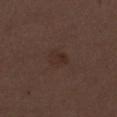The lesion was photographed on a routine skin check and not biopsied; there is no pathology result. Automated tile analysis of the lesion measured an eccentricity of roughly 0.65 and a shape-asymmetry score of about 0.25 (0 = symmetric). And it measured an average lesion color of about L≈28 a*≈16 b*≈21 (CIELAB), roughly 5 lightness units darker than nearby skin, and a normalized border contrast of about 5.5. The software also gave internal color variation of about 2 on a 0–10 scale and a peripheral color-asymmetry measure near 1. The patient is a female approximately 50 years of age. From the left thigh. A close-up tile cropped from a whole-body skin photograph, about 15 mm across.